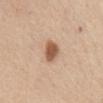Case summary:
- follow-up: catalogued during a skin exam; not biopsied
- image: ~15 mm crop, total-body skin-cancer survey
- patient: female, aged approximately 50
- lesion size: ≈3 mm
- body site: the abdomen
- illumination: white-light